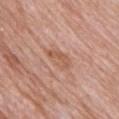Q: Was this lesion biopsied?
A: catalogued during a skin exam; not biopsied
Q: How large is the lesion?
A: ~3.5 mm (longest diameter)
Q: What is the imaging modality?
A: 15 mm crop, total-body photography
Q: Patient demographics?
A: female, approximately 70 years of age
Q: Where on the body is the lesion?
A: the back